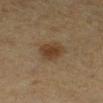The lesion-visualizer software estimated an average lesion color of about L≈32 a*≈14 b*≈26 (CIELAB), roughly 8 lightness units darker than nearby skin, and a lesion-to-skin contrast of about 8 (normalized; higher = more distinct). The software also gave a border-irregularity rating of about 1.5/10, a within-lesion color-variation index near 2.5/10, and peripheral color asymmetry of about 1.
From the right lower leg.
The recorded lesion diameter is about 3 mm.
Captured under cross-polarized illumination.
A female patient approximately 55 years of age.
Cropped from a whole-body photographic skin survey; the tile spans about 15 mm.